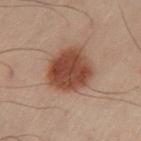Q: Was this lesion biopsied?
A: total-body-photography surveillance lesion; no biopsy